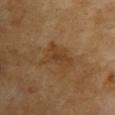notes=no biopsy performed (imaged during a skin exam); patient=female, about 60 years old; site=the chest; image=~15 mm tile from a whole-body skin photo.